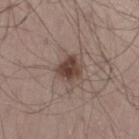Assessment: This lesion was catalogued during total-body skin photography and was not selected for biopsy. Acquisition and patient details: The lesion's longest dimension is about 3 mm. On the left thigh. A male patient in their mid- to late 50s. This is a white-light tile. A lesion tile, about 15 mm wide, cut from a 3D total-body photograph. An algorithmic analysis of the crop reported a footprint of about 7 mm², an outline eccentricity of about 0.5 (0 = round, 1 = elongated), and a shape-asymmetry score of about 0.2 (0 = symmetric). And it measured a lesion color around L≈42 a*≈16 b*≈22 in CIELAB, about 12 CIELAB-L* units darker than the surrounding skin, and a normalized lesion–skin contrast near 9.5. The analysis additionally found a border-irregularity rating of about 2/10, a color-variation rating of about 4/10, and radial color variation of about 1.5.